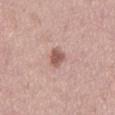The lesion was photographed on a routine skin check and not biopsied; there is no pathology result.
A roughly 15 mm field-of-view crop from a total-body skin photograph.
About 3 mm across.
This is a white-light tile.
From the mid back.
Automated tile analysis of the lesion measured a lesion area of about 4.5 mm² and an outline eccentricity of about 0.8 (0 = round, 1 = elongated). And it measured an average lesion color of about L≈56 a*≈22 b*≈24 (CIELAB), a lesion–skin lightness drop of about 12, and a lesion-to-skin contrast of about 8 (normalized; higher = more distinct). It also reported a border-irregularity rating of about 2.5/10 and a within-lesion color-variation index near 2/10. And it measured lesion-presence confidence of about 100/100.
A male patient aged approximately 45.